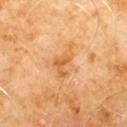Notes:
– follow-up — no biopsy performed (imaged during a skin exam)
– size — about 4 mm
– location — the chest
– image source — ~15 mm tile from a whole-body skin photo
– lighting — cross-polarized illumination
– subject — male, approximately 60 years of age